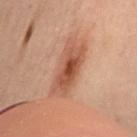follow-up = total-body-photography surveillance lesion; no biopsy
automated metrics = radial color variation of about 1.5
subject = female, aged approximately 55
diameter = ≈6 mm
image = 15 mm crop, total-body photography
anatomic site = the mid back